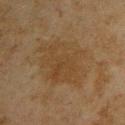Imaged during a routine full-body skin examination; the lesion was not biopsied and no histopathology is available. A male patient, about 45 years old. From the upper back. A 15 mm close-up extracted from a 3D total-body photography capture. Captured under cross-polarized illumination.